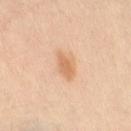Case summary:
- illumination: cross-polarized illumination
- diameter: ~4 mm (longest diameter)
- TBP lesion metrics: a lesion area of about 6 mm² and a symmetry-axis asymmetry near 0.3; a border-irregularity index near 3/10, a color-variation rating of about 1.5/10, and peripheral color asymmetry of about 0.5; a detector confidence of about 100 out of 100 that the crop contains a lesion
- anatomic site: the leg
- patient: female, aged 38 to 42
- imaging modality: 15 mm crop, total-body photography